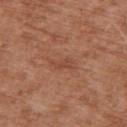workup = catalogued during a skin exam; not biopsied | body site = the right upper arm | illumination = white-light | acquisition = total-body-photography crop, ~15 mm field of view | size = ~3 mm (longest diameter) | patient = male, aged approximately 75.